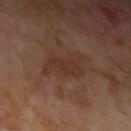Part of a total-body skin-imaging series; this lesion was reviewed on a skin check and was not flagged for biopsy. The lesion-visualizer software estimated a lesion color around L≈32 a*≈18 b*≈26 in CIELAB, roughly 5 lightness units darker than nearby skin, and a normalized border contrast of about 6. The analysis additionally found border irregularity of about 5 on a 0–10 scale, a within-lesion color-variation index near 2/10, and peripheral color asymmetry of about 1. The analysis additionally found an automated nevus-likeness rating near 0 out of 100 and a detector confidence of about 100 out of 100 that the crop contains a lesion. From the arm. A region of skin cropped from a whole-body photographic capture, roughly 15 mm wide. A male patient in their 70s. Captured under cross-polarized illumination.